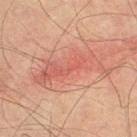This lesion was catalogued during total-body skin photography and was not selected for biopsy. Cropped from a whole-body photographic skin survey; the tile spans about 15 mm. The subject is a male roughly 75 years of age. From the right thigh. Measured at roughly 9 mm in maximum diameter.